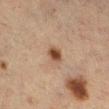Q: Is there a histopathology result?
A: imaged on a skin check; not biopsied
Q: What lighting was used for the tile?
A: cross-polarized illumination
Q: How was this image acquired?
A: ~15 mm tile from a whole-body skin photo
Q: Who is the patient?
A: female, aged around 55
Q: How large is the lesion?
A: ~2.5 mm (longest diameter)
Q: Where on the body is the lesion?
A: the leg
Q: Automated lesion metrics?
A: a border-irregularity index near 1.5/10 and internal color variation of about 2.5 on a 0–10 scale; a classifier nevus-likeness of about 95/100 and lesion-presence confidence of about 100/100